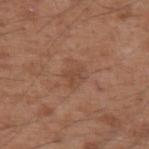Assessment: Imaged during a routine full-body skin examination; the lesion was not biopsied and no histopathology is available. Image and clinical context: Cropped from a total-body skin-imaging series; the visible field is about 15 mm. A male subject aged around 55. The lesion is located on the arm. Automated tile analysis of the lesion measured a footprint of about 5.5 mm² and a shape eccentricity near 0.65. It also reported a border-irregularity rating of about 3/10 and peripheral color asymmetry of about 0.5. Approximately 3.5 mm at its widest. Captured under white-light illumination.